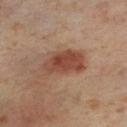The lesion was tiled from a total-body skin photograph and was not biopsied.
Captured under cross-polarized illumination.
A female patient in their mid-50s.
A 15 mm close-up tile from a total-body photography series done for melanoma screening.
Located on the leg.